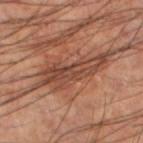- follow-up — catalogued during a skin exam; not biopsied
- anatomic site — the left lower leg
- image-analysis metrics — a footprint of about 19 mm² and a shape eccentricity near 0.95; a lesion color around L≈44 a*≈22 b*≈28 in CIELAB and a normalized border contrast of about 8; a classifier nevus-likeness of about 0/100 and a lesion-detection confidence of about 90/100
- lighting — cross-polarized
- lesion diameter — ≈10 mm
- imaging modality — ~15 mm tile from a whole-body skin photo
- subject — male, approximately 60 years of age